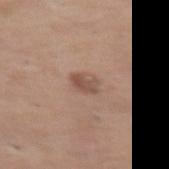The total-body-photography lesion software estimated a mean CIELAB color near L≈51 a*≈19 b*≈26, a lesion–skin lightness drop of about 10, and a normalized border contrast of about 7. And it measured an automated nevus-likeness rating near 55 out of 100.
This is a white-light tile.
Measured at roughly 3 mm in maximum diameter.
A female patient about 65 years old.
A roughly 15 mm field-of-view crop from a total-body skin photograph.
On the front of the torso.